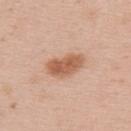Part of a total-body skin-imaging series; this lesion was reviewed on a skin check and was not flagged for biopsy.